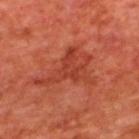No biopsy was performed on this lesion — it was imaged during a full skin examination and was not determined to be concerning.
This is a cross-polarized tile.
About 7 mm across.
Cropped from a total-body skin-imaging series; the visible field is about 15 mm.
A male patient, in their mid- to late 60s.
The lesion is on the upper back.